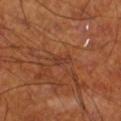biopsy status = catalogued during a skin exam; not biopsied
subject = male, aged 68 to 72
site = the right lower leg
imaging modality = ~15 mm crop, total-body skin-cancer survey